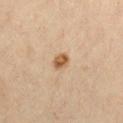No biopsy was performed on this lesion — it was imaged during a full skin examination and was not determined to be concerning. A female subject in their 30s. A 15 mm close-up tile from a total-body photography series done for melanoma screening. About 2 mm across. Located on the chest. Captured under cross-polarized illumination.